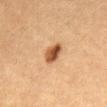Part of a total-body skin-imaging series; this lesion was reviewed on a skin check and was not flagged for biopsy. The lesion is located on the abdomen. A female patient, aged 58–62. This image is a 15 mm lesion crop taken from a total-body photograph.